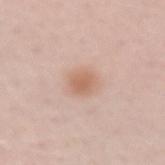Q: Was this lesion biopsied?
A: no biopsy performed (imaged during a skin exam)
Q: Patient demographics?
A: female, aged 43 to 47
Q: How large is the lesion?
A: about 3 mm
Q: What lighting was used for the tile?
A: white-light illumination
Q: How was this image acquired?
A: 15 mm crop, total-body photography
Q: Lesion location?
A: the left forearm